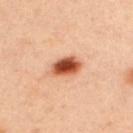Imaged during a routine full-body skin examination; the lesion was not biopsied and no histopathology is available.
The subject is a male aged approximately 35.
A roughly 15 mm field-of-view crop from a total-body skin photograph.
The lesion is located on the upper back.